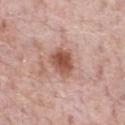Notes:
* follow-up · total-body-photography surveillance lesion; no biopsy
* image · 15 mm crop, total-body photography
* tile lighting · white-light illumination
* lesion diameter · ≈3.5 mm
* patient · male, aged around 70
* location · the chest